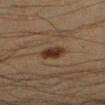automated metrics = a lesion area of about 5.5 mm² and two-axis asymmetry of about 0.15; a classifier nevus-likeness of about 95/100 and lesion-presence confidence of about 100/100 | acquisition = 15 mm crop, total-body photography | anatomic site = the right forearm | lighting = cross-polarized | patient = male, aged approximately 35.